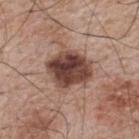workup: catalogued during a skin exam; not biopsied
illumination: white-light
patient: male, aged 63–67
lesion diameter: ~5 mm (longest diameter)
automated metrics: an area of roughly 17 mm², an outline eccentricity of about 0.5 (0 = round, 1 = elongated), and a symmetry-axis asymmetry near 0.2; a mean CIELAB color near L≈43 a*≈20 b*≈24, a lesion–skin lightness drop of about 17, and a lesion-to-skin contrast of about 12.5 (normalized; higher = more distinct); a classifier nevus-likeness of about 50/100 and lesion-presence confidence of about 100/100
body site: the upper back
imaging modality: total-body-photography crop, ~15 mm field of view